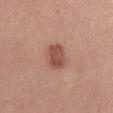- location: the chest
- automated lesion analysis: an average lesion color of about L≈51 a*≈24 b*≈27 (CIELAB), a lesion–skin lightness drop of about 12, and a lesion-to-skin contrast of about 8 (normalized; higher = more distinct)
- acquisition: 15 mm crop, total-body photography
- subject: male, in their mid- to late 20s